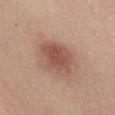Assessment: Part of a total-body skin-imaging series; this lesion was reviewed on a skin check and was not flagged for biopsy. Acquisition and patient details: The patient is a female roughly 30 years of age. From the chest. An algorithmic analysis of the crop reported an eccentricity of roughly 0.8 and a symmetry-axis asymmetry near 0.2. The software also gave roughly 10 lightness units darker than nearby skin. It also reported a detector confidence of about 100 out of 100 that the crop contains a lesion. This is a white-light tile. A 15 mm crop from a total-body photograph taken for skin-cancer surveillance. Measured at roughly 5.5 mm in maximum diameter.